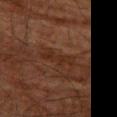<record>
<biopsy_status>not biopsied; imaged during a skin examination</biopsy_status>
<lighting>cross-polarized</lighting>
<lesion_size>
  <long_diameter_mm_approx>4.0</long_diameter_mm_approx>
</lesion_size>
<automated_metrics>
  <area_mm2_approx>5.0</area_mm2_approx>
  <eccentricity>0.95</eccentricity>
  <shape_asymmetry>0.35</shape_asymmetry>
  <cielab_L>21</cielab_L>
  <cielab_a>16</cielab_a>
  <cielab_b>22</cielab_b>
  <vs_skin_darker_L>4.0</vs_skin_darker_L>
  <vs_skin_contrast_norm>6.0</vs_skin_contrast_norm>
  <nevus_likeness_0_100>0</nevus_likeness_0_100>
  <lesion_detection_confidence_0_100>80</lesion_detection_confidence_0_100>
</automated_metrics>
<site>left thigh</site>
<image>
  <source>total-body photography crop</source>
  <field_of_view_mm>15</field_of_view_mm>
</image>
<patient>
  <sex>male</sex>
  <age_approx>80</age_approx>
</patient>
</record>